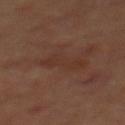Case summary:
* follow-up — total-body-photography surveillance lesion; no biopsy
* acquisition — total-body-photography crop, ~15 mm field of view
* body site — the mid back
* illumination — cross-polarized illumination
* size — ≈4.5 mm
* subject — male, approximately 70 years of age